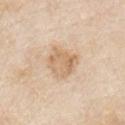Q: Was this lesion biopsied?
A: total-body-photography surveillance lesion; no biopsy
Q: How was the tile lit?
A: white-light illumination
Q: What is the imaging modality?
A: ~15 mm tile from a whole-body skin photo
Q: Patient demographics?
A: male, roughly 80 years of age
Q: Where on the body is the lesion?
A: the chest
Q: Lesion size?
A: ~3.5 mm (longest diameter)
Q: Automated lesion metrics?
A: an area of roughly 10 mm², a shape eccentricity near 0.4, and a shape-asymmetry score of about 0.25 (0 = symmetric); a border-irregularity index near 2.5/10, a color-variation rating of about 4/10, and radial color variation of about 1.5; an automated nevus-likeness rating near 5 out of 100 and a lesion-detection confidence of about 100/100